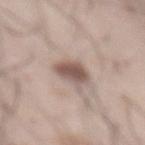biopsy status=catalogued during a skin exam; not biopsied | lesion size=≈3.5 mm | site=the abdomen | image=total-body-photography crop, ~15 mm field of view | patient=male, aged around 55.